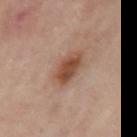Imaged during a routine full-body skin examination; the lesion was not biopsied and no histopathology is available. On the left upper arm. A subject aged 53–57. This is a cross-polarized tile. The total-body-photography lesion software estimated a footprint of about 9 mm², a shape eccentricity near 0.7, and two-axis asymmetry of about 0.2. And it measured a mean CIELAB color near L≈48 a*≈20 b*≈29 and a lesion–skin lightness drop of about 11. It also reported a nevus-likeness score of about 85/100 and lesion-presence confidence of about 100/100. Approximately 4 mm at its widest. This image is a 15 mm lesion crop taken from a total-body photograph.